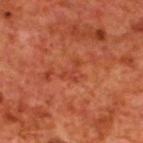Q: Is there a histopathology result?
A: total-body-photography surveillance lesion; no biopsy
Q: What are the patient's age and sex?
A: male, approximately 70 years of age
Q: Automated lesion metrics?
A: a lesion area of about 2.5 mm², an eccentricity of roughly 0.8, and two-axis asymmetry of about 0.8; a border-irregularity rating of about 8/10 and peripheral color asymmetry of about 0; a nevus-likeness score of about 0/100 and a lesion-detection confidence of about 100/100
Q: What kind of image is this?
A: ~15 mm crop, total-body skin-cancer survey
Q: What is the anatomic site?
A: the upper back